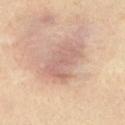workup = total-body-photography surveillance lesion; no biopsy | anatomic site = the upper back | subject = male, in their mid- to late 30s | TBP lesion metrics = an area of roughly 11 mm² and an eccentricity of roughly 0.8; an automated nevus-likeness rating near 0 out of 100 and lesion-presence confidence of about 100/100 | diameter = ≈4.5 mm | acquisition = 15 mm crop, total-body photography | illumination = cross-polarized illumination.